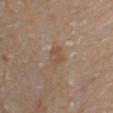Q: Is there a histopathology result?
A: catalogued during a skin exam; not biopsied
Q: Patient demographics?
A: male, aged 78 to 82
Q: How large is the lesion?
A: ≈2.5 mm
Q: What is the anatomic site?
A: the left upper arm
Q: What is the imaging modality?
A: 15 mm crop, total-body photography